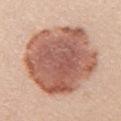Impression:
This lesion was catalogued during total-body skin photography and was not selected for biopsy.
Image and clinical context:
The tile uses white-light illumination. Automated image analysis of the tile measured a shape eccentricity near 0.55 and two-axis asymmetry of about 0.15. It also reported a mean CIELAB color near L≈58 a*≈23 b*≈28, roughly 17 lightness units darker than nearby skin, and a normalized lesion–skin contrast near 10.5. And it measured internal color variation of about 6 on a 0–10 scale. Approximately 9.5 mm at its widest. The lesion is located on the right upper arm. A lesion tile, about 15 mm wide, cut from a 3D total-body photograph. The patient is a female roughly 25 years of age.